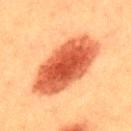Clinical impression: This lesion was catalogued during total-body skin photography and was not selected for biopsy. Acquisition and patient details: Imaged with cross-polarized lighting. This image is a 15 mm lesion crop taken from a total-body photograph. A male subject, approximately 40 years of age. Longest diameter approximately 10 mm. The lesion is located on the upper back.